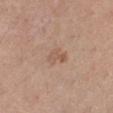| feature | finding |
|---|---|
| biopsy status | catalogued during a skin exam; not biopsied |
| image source | 15 mm crop, total-body photography |
| automated metrics | a border-irregularity index near 4/10, a within-lesion color-variation index near 2.5/10, and radial color variation of about 0.5 |
| body site | the right lower leg |
| illumination | white-light |
| subject | female, in their mid- to late 40s |
| size | ~3 mm (longest diameter) |